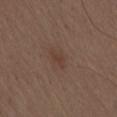notes=catalogued during a skin exam; not biopsied | tile lighting=white-light illumination | location=the lower back | size=about 2.5 mm | subject=male, in their 70s | imaging modality=~15 mm tile from a whole-body skin photo.